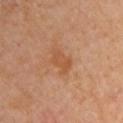Imaged during a routine full-body skin examination; the lesion was not biopsied and no histopathology is available. A 15 mm close-up tile from a total-body photography series done for melanoma screening. The total-body-photography lesion software estimated a footprint of about 6 mm² and a shape eccentricity near 0.85. It also reported a lesion color around L≈51 a*≈23 b*≈35 in CIELAB, roughly 7 lightness units darker than nearby skin, and a normalized border contrast of about 6. The analysis additionally found a border-irregularity rating of about 4/10, internal color variation of about 2 on a 0–10 scale, and peripheral color asymmetry of about 0.5. The analysis additionally found a nevus-likeness score of about 10/100 and a lesion-detection confidence of about 100/100. A female subject, approximately 50 years of age. The tile uses cross-polarized illumination. Longest diameter approximately 4 mm. Located on the upper back.